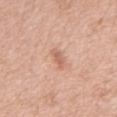Assessment:
The lesion was tiled from a total-body skin photograph and was not biopsied.
Acquisition and patient details:
A 15 mm close-up extracted from a 3D total-body photography capture. Automated tile analysis of the lesion measured about 9 CIELAB-L* units darker than the surrounding skin and a normalized lesion–skin contrast near 6. The analysis additionally found a border-irregularity index near 4/10 and internal color variation of about 1.5 on a 0–10 scale. The analysis additionally found a classifier nevus-likeness of about 0/100 and a detector confidence of about 100 out of 100 that the crop contains a lesion. Measured at roughly 3 mm in maximum diameter. A male subject approximately 50 years of age. Captured under white-light illumination. On the abdomen.